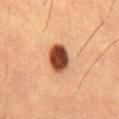Impression:
Captured during whole-body skin photography for melanoma surveillance; the lesion was not biopsied.
Context:
The lesion-visualizer software estimated a color-variation rating of about 6/10 and a peripheral color-asymmetry measure near 2. It also reported a nevus-likeness score of about 100/100 and a detector confidence of about 100 out of 100 that the crop contains a lesion. A 15 mm close-up extracted from a 3D total-body photography capture. Located on the abdomen. The patient is a male aged around 55. Captured under cross-polarized illumination.